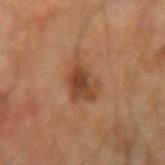Imaged during a routine full-body skin examination; the lesion was not biopsied and no histopathology is available.
The lesion's longest dimension is about 4.5 mm.
A male patient aged 63 to 67.
A 15 mm crop from a total-body photograph taken for skin-cancer surveillance.
From the left forearm.